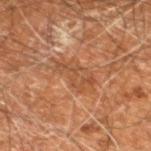| key | value |
|---|---|
| notes | no biopsy performed (imaged during a skin exam) |
| patient | male, aged 58 to 62 |
| image source | ~15 mm crop, total-body skin-cancer survey |
| site | the leg |
| illumination | cross-polarized illumination |
| size | ≈5.5 mm |